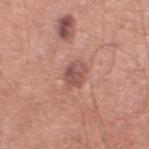workup = no biopsy performed (imaged during a skin exam)
lesion size = ~3 mm (longest diameter)
image source = 15 mm crop, total-body photography
location = the leg
subject = male, roughly 70 years of age
illumination = white-light illumination